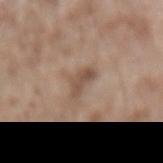No biopsy was performed on this lesion — it was imaged during a full skin examination and was not determined to be concerning.
A 15 mm close-up tile from a total-body photography series done for melanoma screening.
Imaged with white-light lighting.
The lesion is located on the lower back.
An algorithmic analysis of the crop reported a mean CIELAB color near L≈50 a*≈16 b*≈27 and a normalized lesion–skin contrast near 7. The analysis additionally found a border-irregularity rating of about 4/10, internal color variation of about 3 on a 0–10 scale, and a peripheral color-asymmetry measure near 1. The analysis additionally found an automated nevus-likeness rating near 0 out of 100 and lesion-presence confidence of about 100/100.
The patient is a male approximately 55 years of age.
About 3 mm across.